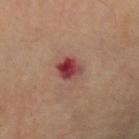Assessment:
The lesion was photographed on a routine skin check and not biopsied; there is no pathology result.
Image and clinical context:
A 15 mm crop from a total-body photograph taken for skin-cancer surveillance. The subject is a male roughly 70 years of age. Captured under cross-polarized illumination. Measured at roughly 3 mm in maximum diameter. The lesion is on the left forearm.